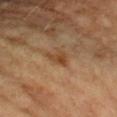– notes: imaged on a skin check; not biopsied
– location: the left forearm
– image source: ~15 mm tile from a whole-body skin photo
– patient: female, aged around 60
– automated metrics: a border-irregularity rating of about 5/10 and internal color variation of about 2.5 on a 0–10 scale